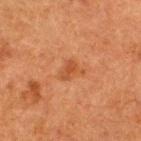Q: Is there a histopathology result?
A: no biopsy performed (imaged during a skin exam)
Q: What kind of image is this?
A: ~15 mm crop, total-body skin-cancer survey
Q: What is the lesion's diameter?
A: ~3 mm (longest diameter)
Q: Patient demographics?
A: male, roughly 50 years of age
Q: What is the anatomic site?
A: the upper back
Q: How was the tile lit?
A: cross-polarized